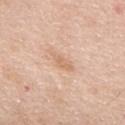workup: no biopsy performed (imaged during a skin exam); image: ~15 mm crop, total-body skin-cancer survey; image-analysis metrics: a lesion area of about 3.5 mm², an eccentricity of roughly 0.9, and a symmetry-axis asymmetry near 0.4; patient: male, about 75 years old; size: ≈3 mm; location: the upper back.